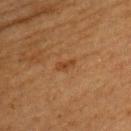biopsy_status: not biopsied; imaged during a skin examination
image:
  source: total-body photography crop
  field_of_view_mm: 15
lesion_size:
  long_diameter_mm_approx: 2.5
automated_metrics:
  area_mm2_approx: 3.0
  shape_asymmetry: 0.3
  cielab_L: 37
  cielab_a: 20
  cielab_b: 32
  vs_skin_darker_L: 6.0
  vs_skin_contrast_norm: 6.0
  nevus_likeness_0_100: 35
  lesion_detection_confidence_0_100: 100
lighting: cross-polarized
site: left upper arm
patient:
  sex: female
  age_approx: 50